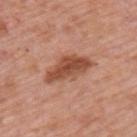Findings:
• follow-up · imaged on a skin check; not biopsied
• subject · male, aged around 75
• lesion diameter · about 6 mm
• illumination · white-light illumination
• image source · ~15 mm crop, total-body skin-cancer survey
• automated lesion analysis · a mean CIELAB color near L≈50 a*≈25 b*≈32 and a normalized lesion–skin contrast near 9; a nevus-likeness score of about 85/100 and lesion-presence confidence of about 100/100
• site · the upper back